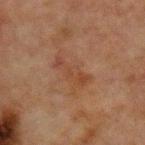Assessment:
No biopsy was performed on this lesion — it was imaged during a full skin examination and was not determined to be concerning.
Acquisition and patient details:
The lesion is on the chest. This is a cross-polarized tile. A 15 mm close-up extracted from a 3D total-body photography capture. A male patient, about 65 years old.